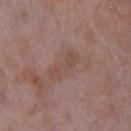Impression: This lesion was catalogued during total-body skin photography and was not selected for biopsy. Context: Cropped from a whole-body photographic skin survey; the tile spans about 15 mm. About 4.5 mm across. Located on the right lower leg. Captured under white-light illumination. The patient is a female approximately 70 years of age. The total-body-photography lesion software estimated a within-lesion color-variation index near 2/10 and radial color variation of about 0.5.